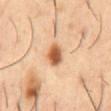No biopsy was performed on this lesion — it was imaged during a full skin examination and was not determined to be concerning.
A male patient, aged around 55.
Measured at roughly 3.5 mm in maximum diameter.
The total-body-photography lesion software estimated an average lesion color of about L≈58 a*≈24 b*≈38 (CIELAB), about 16 CIELAB-L* units darker than the surrounding skin, and a normalized lesion–skin contrast near 10.5. And it measured border irregularity of about 1.5 on a 0–10 scale, internal color variation of about 5 on a 0–10 scale, and peripheral color asymmetry of about 1.5. And it measured an automated nevus-likeness rating near 100 out of 100 and a lesion-detection confidence of about 100/100.
A 15 mm close-up extracted from a 3D total-body photography capture.
Captured under cross-polarized illumination.
Located on the abdomen.